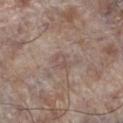Clinical summary: Longest diameter approximately 2.5 mm. Cropped from a total-body skin-imaging series; the visible field is about 15 mm. The subject is a male approximately 70 years of age. The lesion is on the left lower leg. This is a white-light tile.